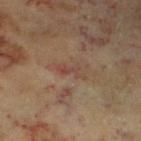Q: Is there a histopathology result?
A: imaged on a skin check; not biopsied
Q: What is the imaging modality?
A: total-body-photography crop, ~15 mm field of view
Q: Where on the body is the lesion?
A: the left lower leg
Q: Patient demographics?
A: female, about 60 years old
Q: How large is the lesion?
A: about 3 mm